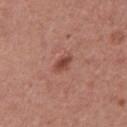Impression:
Captured during whole-body skin photography for melanoma surveillance; the lesion was not biopsied.
Context:
The lesion is located on the upper back. A male patient, aged 43–47. Cropped from a total-body skin-imaging series; the visible field is about 15 mm. Automated tile analysis of the lesion measured a lesion area of about 3.5 mm² and a shape eccentricity near 0.8. It also reported a lesion color around L≈46 a*≈25 b*≈28 in CIELAB, about 10 CIELAB-L* units darker than the surrounding skin, and a lesion-to-skin contrast of about 8 (normalized; higher = more distinct). And it measured a border-irregularity index near 2/10, a within-lesion color-variation index near 2/10, and peripheral color asymmetry of about 0.5. It also reported an automated nevus-likeness rating near 80 out of 100 and lesion-presence confidence of about 100/100. Captured under white-light illumination.